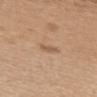workup: imaged on a skin check; not biopsied | image source: 15 mm crop, total-body photography | patient: female, aged around 60 | diameter: ~2.5 mm (longest diameter) | site: the upper back.